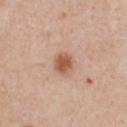subject = male, in their 60s | acquisition = 15 mm crop, total-body photography | site = the chest | image-analysis metrics = an eccentricity of roughly 0.5 and two-axis asymmetry of about 0.15; a lesion-detection confidence of about 100/100.